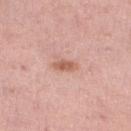Captured during whole-body skin photography for melanoma surveillance; the lesion was not biopsied.
About 3 mm across.
A male patient, roughly 60 years of age.
This image is a 15 mm lesion crop taken from a total-body photograph.
The lesion is on the right lower leg.